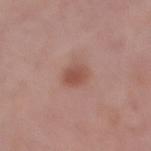The lesion was tiled from a total-body skin photograph and was not biopsied. The lesion is located on the right thigh. A close-up tile cropped from a whole-body skin photograph, about 15 mm across. The lesion's longest dimension is about 3 mm. Imaged with white-light lighting. Automated image analysis of the tile measured a mean CIELAB color near L≈51 a*≈23 b*≈27, about 9 CIELAB-L* units darker than the surrounding skin, and a lesion-to-skin contrast of about 7 (normalized; higher = more distinct). It also reported a classifier nevus-likeness of about 85/100 and a detector confidence of about 100 out of 100 that the crop contains a lesion. A female patient in their 50s.